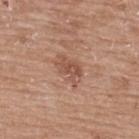Q: Was a biopsy performed?
A: total-body-photography surveillance lesion; no biopsy
Q: What did automated image analysis measure?
A: an area of roughly 6 mm², an outline eccentricity of about 0.8 (0 = round, 1 = elongated), and a symmetry-axis asymmetry near 0.4
Q: Lesion size?
A: ≈3.5 mm
Q: What is the anatomic site?
A: the upper back
Q: Illumination type?
A: white-light
Q: What is the imaging modality?
A: total-body-photography crop, ~15 mm field of view
Q: What are the patient's age and sex?
A: female, aged 58 to 62